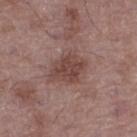follow-up: total-body-photography surveillance lesion; no biopsy | site: the left thigh | lighting: white-light illumination | diameter: about 4.5 mm | patient: male, roughly 50 years of age | image: 15 mm crop, total-body photography | automated metrics: a shape eccentricity near 0.7 and a shape-asymmetry score of about 0.2 (0 = symmetric); a lesion color around L≈44 a*≈19 b*≈22 in CIELAB and a lesion-to-skin contrast of about 7.5 (normalized; higher = more distinct); internal color variation of about 3 on a 0–10 scale and radial color variation of about 1.